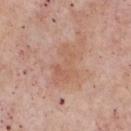<record>
  <biopsy_status>not biopsied; imaged during a skin examination</biopsy_status>
  <patient>
    <sex>male</sex>
    <age_approx>55</age_approx>
  </patient>
  <lighting>white-light</lighting>
  <image>
    <source>total-body photography crop</source>
    <field_of_view_mm>15</field_of_view_mm>
  </image>
  <automated_metrics>
    <area_mm2_approx>11.0</area_mm2_approx>
    <eccentricity>0.85</eccentricity>
    <shape_asymmetry>0.4</shape_asymmetry>
    <cielab_L>59</cielab_L>
    <cielab_a>21</cielab_a>
    <cielab_b>30</cielab_b>
    <vs_skin_darker_L>6.0</vs_skin_darker_L>
  </automated_metrics>
  <lesion_size>
    <long_diameter_mm_approx>5.0</long_diameter_mm_approx>
  </lesion_size>
  <site>chest</site>
</record>